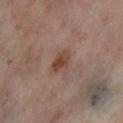biopsy status: no biopsy performed (imaged during a skin exam) | automated lesion analysis: a shape eccentricity near 0.8 and a shape-asymmetry score of about 0.3 (0 = symmetric); a lesion color around L≈43 a*≈19 b*≈27 in CIELAB, roughly 10 lightness units darker than nearby skin, and a normalized border contrast of about 8.5; border irregularity of about 2.5 on a 0–10 scale, a color-variation rating of about 3.5/10, and radial color variation of about 1 | image source: ~15 mm crop, total-body skin-cancer survey | illumination: cross-polarized | anatomic site: the leg | subject: female, about 55 years old.